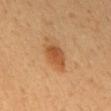Case summary:
– lighting: cross-polarized illumination
– diameter: ~4 mm (longest diameter)
– automated lesion analysis: a shape eccentricity near 0.8 and a shape-asymmetry score of about 0.2 (0 = symmetric); a mean CIELAB color near L≈49 a*≈22 b*≈37 and a lesion-to-skin contrast of about 7.5 (normalized; higher = more distinct); a nevus-likeness score of about 95/100 and a detector confidence of about 100 out of 100 that the crop contains a lesion
– body site: the mid back
– patient: male, roughly 45 years of age
– image: ~15 mm tile from a whole-body skin photo